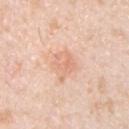Clinical impression:
Captured during whole-body skin photography for melanoma surveillance; the lesion was not biopsied.
Clinical summary:
A male subject, aged 48–52. The lesion is located on the chest. A 15 mm close-up extracted from a 3D total-body photography capture. The recorded lesion diameter is about 3.5 mm. An algorithmic analysis of the crop reported an average lesion color of about L≈72 a*≈22 b*≈32 (CIELAB), a lesion–skin lightness drop of about 8, and a normalized border contrast of about 5. And it measured a nevus-likeness score of about 10/100 and a detector confidence of about 100 out of 100 that the crop contains a lesion.